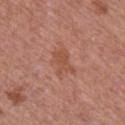Assessment:
This lesion was catalogued during total-body skin photography and was not selected for biopsy.
Acquisition and patient details:
The patient is a male aged around 65. This is a white-light tile. The lesion-visualizer software estimated an area of roughly 6 mm², an eccentricity of roughly 0.75, and a symmetry-axis asymmetry near 0.3. The analysis additionally found a border-irregularity rating of about 3.5/10. Measured at roughly 3.5 mm in maximum diameter. On the right upper arm. A region of skin cropped from a whole-body photographic capture, roughly 15 mm wide.